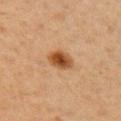Impression: The lesion was tiled from a total-body skin photograph and was not biopsied. Context: A region of skin cropped from a whole-body photographic capture, roughly 15 mm wide. This is a cross-polarized tile. Measured at roughly 3 mm in maximum diameter. The lesion-visualizer software estimated an average lesion color of about L≈42 a*≈20 b*≈34 (CIELAB) and a lesion-to-skin contrast of about 10 (normalized; higher = more distinct). It also reported an automated nevus-likeness rating near 100 out of 100 and a lesion-detection confidence of about 100/100. The lesion is on the arm. A female subject, about 40 years old.